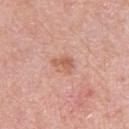follow-up — catalogued during a skin exam; not biopsied | site — the arm | image-analysis metrics — a footprint of about 4.5 mm², an eccentricity of roughly 0.7, and a symmetry-axis asymmetry near 0.25; a classifier nevus-likeness of about 5/100 and a detector confidence of about 100 out of 100 that the crop contains a lesion | size — ~3 mm (longest diameter) | acquisition — total-body-photography crop, ~15 mm field of view | patient — male, aged approximately 80 | lighting — white-light.